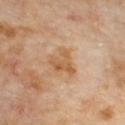patient: male, in their 70s; imaging modality: ~15 mm tile from a whole-body skin photo; location: the chest.